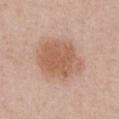The recorded lesion diameter is about 6 mm. From the chest. An algorithmic analysis of the crop reported a footprint of about 21 mm², an outline eccentricity of about 0.6 (0 = round, 1 = elongated), and a shape-asymmetry score of about 0.15 (0 = symmetric). And it measured a border-irregularity index near 1.5/10, a within-lesion color-variation index near 3/10, and a peripheral color-asymmetry measure near 1. It also reported a nevus-likeness score of about 100/100. A female patient, roughly 40 years of age. A lesion tile, about 15 mm wide, cut from a 3D total-body photograph. Captured under white-light illumination.